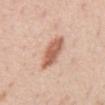{
  "biopsy_status": "not biopsied; imaged during a skin examination",
  "patient": {
    "sex": "male",
    "age_approx": 55
  },
  "lighting": "white-light",
  "lesion_size": {
    "long_diameter_mm_approx": 4.5
  },
  "image": {
    "source": "total-body photography crop",
    "field_of_view_mm": 15
  },
  "site": "back"
}